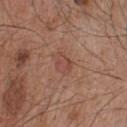Background:
On the back. A 15 mm crop from a total-body photograph taken for skin-cancer surveillance. A male subject roughly 45 years of age.